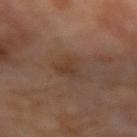The lesion was photographed on a routine skin check and not biopsied; there is no pathology result. The tile uses cross-polarized illumination. A region of skin cropped from a whole-body photographic capture, roughly 15 mm wide. Longest diameter approximately 2.5 mm. A male subject aged approximately 70. The lesion is on the arm.